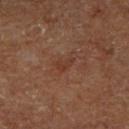Q: Was a biopsy performed?
A: no biopsy performed (imaged during a skin exam)
Q: How was this image acquired?
A: 15 mm crop, total-body photography
Q: Where on the body is the lesion?
A: the right lower leg
Q: What are the patient's age and sex?
A: male, in their 60s
Q: How was the tile lit?
A: cross-polarized
Q: How large is the lesion?
A: ≈2.5 mm